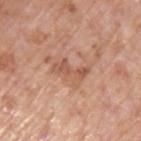Recorded during total-body skin imaging; not selected for excision or biopsy.
The lesion is located on the left upper arm.
Approximately 4 mm at its widest.
This is a white-light tile.
A male patient aged 68–72.
This image is a 15 mm lesion crop taken from a total-body photograph.